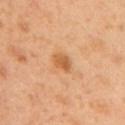This lesion was catalogued during total-body skin photography and was not selected for biopsy. The lesion is located on the left upper arm. A 15 mm close-up tile from a total-body photography series done for melanoma screening. A female subject, aged approximately 40.